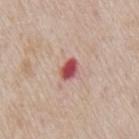<lesion>
<biopsy_status>not biopsied; imaged during a skin examination</biopsy_status>
<lighting>white-light</lighting>
<patient>
  <sex>male</sex>
  <age_approx>65</age_approx>
</patient>
<image>
  <source>total-body photography crop</source>
  <field_of_view_mm>15</field_of_view_mm>
</image>
<lesion_size>
  <long_diameter_mm_approx>3.0</long_diameter_mm_approx>
</lesion_size>
<site>mid back</site>
<automated_metrics>
  <area_mm2_approx>4.5</area_mm2_approx>
  <eccentricity>0.7</eccentricity>
  <shape_asymmetry>0.25</shape_asymmetry>
</automated_metrics>
</lesion>